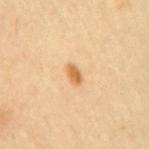Case summary:
• biopsy status · total-body-photography surveillance lesion; no biopsy
• size · ~2.5 mm (longest diameter)
• image · total-body-photography crop, ~15 mm field of view
• tile lighting · cross-polarized
• patient · female, in their mid- to late 50s
• TBP lesion metrics · an area of roughly 3 mm² and a symmetry-axis asymmetry near 0.25; a border-irregularity rating of about 2/10 and internal color variation of about 1.5 on a 0–10 scale; a nevus-likeness score of about 95/100 and a detector confidence of about 100 out of 100 that the crop contains a lesion
• body site · the mid back